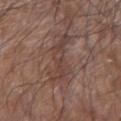<case>
  <biopsy_status>not biopsied; imaged during a skin examination</biopsy_status>
  <patient>
    <sex>male</sex>
    <age_approx>80</age_approx>
  </patient>
  <lighting>white-light</lighting>
  <image>
    <source>total-body photography crop</source>
    <field_of_view_mm>15</field_of_view_mm>
  </image>
  <lesion_size>
    <long_diameter_mm_approx>5.5</long_diameter_mm_approx>
  </lesion_size>
  <site>arm</site>
</case>